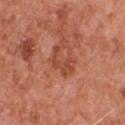{"biopsy_status": "not biopsied; imaged during a skin examination", "patient": {"sex": "male", "age_approx": 55}, "lighting": "white-light", "image": {"source": "total-body photography crop", "field_of_view_mm": 15}, "automated_metrics": {"cielab_L": 48, "cielab_a": 30, "cielab_b": 34, "vs_skin_darker_L": 8.0, "vs_skin_contrast_norm": 6.0, "border_irregularity_0_10": 7.0, "color_variation_0_10": 1.5, "peripheral_color_asymmetry": 0.5}, "lesion_size": {"long_diameter_mm_approx": 4.0}, "site": "chest"}